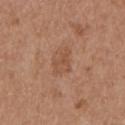<lesion>
<biopsy_status>not biopsied; imaged during a skin examination</biopsy_status>
<patient>
  <sex>male</sex>
  <age_approx>55</age_approx>
</patient>
<lesion_size>
  <long_diameter_mm_approx>3.0</long_diameter_mm_approx>
</lesion_size>
<site>left upper arm</site>
<lighting>white-light</lighting>
<automated_metrics>
  <eccentricity>0.75</eccentricity>
  <cielab_L>51</cielab_L>
  <cielab_a>22</cielab_a>
  <cielab_b>31</cielab_b>
  <vs_skin_darker_L>7.0</vs_skin_darker_L>
  <vs_skin_contrast_norm>5.0</vs_skin_contrast_norm>
  <border_irregularity_0_10>4.0</border_irregularity_0_10>
  <peripheral_color_asymmetry>0.5</peripheral_color_asymmetry>
  <nevus_likeness_0_100>0</nevus_likeness_0_100>
</automated_metrics>
<image>
  <source>total-body photography crop</source>
  <field_of_view_mm>15</field_of_view_mm>
</image>
</lesion>